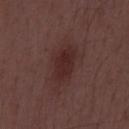Q: Was a biopsy performed?
A: imaged on a skin check; not biopsied
Q: Lesion size?
A: ≈5.5 mm
Q: What lighting was used for the tile?
A: white-light illumination
Q: Who is the patient?
A: male, in their 50s
Q: What kind of image is this?
A: ~15 mm crop, total-body skin-cancer survey